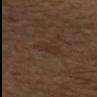biopsy status: imaged on a skin check; not biopsied
location: the arm
subject: male, roughly 60 years of age
image: 15 mm crop, total-body photography